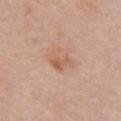No biopsy was performed on this lesion — it was imaged during a full skin examination and was not determined to be concerning. A lesion tile, about 15 mm wide, cut from a 3D total-body photograph. A female patient aged 63 to 67. From the chest. Longest diameter approximately 3.5 mm. Automated image analysis of the tile measured an eccentricity of roughly 0.7 and a symmetry-axis asymmetry near 0.35. The software also gave a lesion–skin lightness drop of about 7 and a normalized border contrast of about 6. It also reported border irregularity of about 4.5 on a 0–10 scale, internal color variation of about 3 on a 0–10 scale, and radial color variation of about 1.